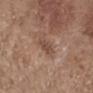The recorded lesion diameter is about 3 mm. The lesion is located on the right forearm. A region of skin cropped from a whole-body photographic capture, roughly 15 mm wide. The patient is a female aged 63 to 67.